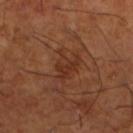image source — ~15 mm crop, total-body skin-cancer survey
subject — male, approximately 65 years of age
size — ≈3.5 mm
lighting — cross-polarized
body site — the leg